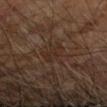follow-up: catalogued during a skin exam; not biopsied
anatomic site: the right forearm
image: 15 mm crop, total-body photography
patient: male, roughly 70 years of age